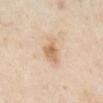Case summary:
– notes · no biopsy performed (imaged during a skin exam)
– automated lesion analysis · a footprint of about 5 mm², an eccentricity of roughly 0.8, and two-axis asymmetry of about 0.3; border irregularity of about 3 on a 0–10 scale, a color-variation rating of about 3/10, and radial color variation of about 1
– illumination · cross-polarized illumination
– image · total-body-photography crop, ~15 mm field of view
– anatomic site · the chest
– subject · male, in their mid-60s
– size · ≈3.5 mm